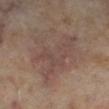Q: What kind of image is this?
A: ~15 mm tile from a whole-body skin photo
Q: How was the tile lit?
A: cross-polarized illumination
Q: Lesion size?
A: about 8 mm
Q: Lesion location?
A: the leg
Q: Patient demographics?
A: female, in their 60s